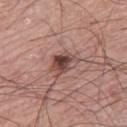{"biopsy_status": "not biopsied; imaged during a skin examination", "patient": {"sex": "male", "age_approx": 75}, "image": {"source": "total-body photography crop", "field_of_view_mm": 15}, "lesion_size": {"long_diameter_mm_approx": 3.5}, "site": "leg"}